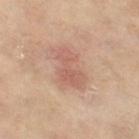Imaged during a routine full-body skin examination; the lesion was not biopsied and no histopathology is available.
A close-up tile cropped from a whole-body skin photograph, about 15 mm across.
This is a cross-polarized tile.
The lesion is on the left thigh.
A female patient roughly 75 years of age.
About 5.5 mm across.